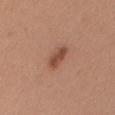biopsy status = no biopsy performed (imaged during a skin exam) | body site = the head or neck | subject = female, in their mid- to late 40s | lesion size = ~3.5 mm (longest diameter) | image = total-body-photography crop, ~15 mm field of view.